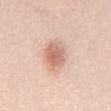<lesion>
  <biopsy_status>not biopsied; imaged during a skin examination</biopsy_status>
</lesion>